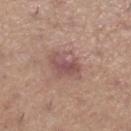<lesion>
<biopsy_status>not biopsied; imaged during a skin examination</biopsy_status>
<image>
  <source>total-body photography crop</source>
  <field_of_view_mm>15</field_of_view_mm>
</image>
<site>right lower leg</site>
<lighting>white-light</lighting>
<automated_metrics>
  <area_mm2_approx>10.0</area_mm2_approx>
  <eccentricity>0.6</eccentricity>
  <shape_asymmetry>0.25</shape_asymmetry>
  <cielab_L>52</cielab_L>
  <cielab_a>21</cielab_a>
  <cielab_b>21</cielab_b>
  <vs_skin_darker_L>9.0</vs_skin_darker_L>
  <vs_skin_contrast_norm>7.0</vs_skin_contrast_norm>
  <border_irregularity_0_10>3.0</border_irregularity_0_10>
  <color_variation_0_10>5.0</color_variation_0_10>
  <peripheral_color_asymmetry>1.5</peripheral_color_asymmetry>
</automated_metrics>
<lesion_size>
  <long_diameter_mm_approx>4.0</long_diameter_mm_approx>
</lesion_size>
<patient>
  <sex>female</sex>
  <age_approx>45</age_approx>
</patient>
</lesion>